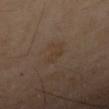Clinical impression: This lesion was catalogued during total-body skin photography and was not selected for biopsy.